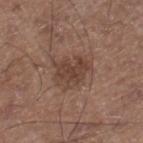subject = male, roughly 65 years of age
imaging modality = total-body-photography crop, ~15 mm field of view
lighting = white-light illumination
diameter = about 4.5 mm
anatomic site = the right lower leg